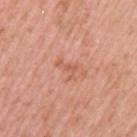Part of a total-body skin-imaging series; this lesion was reviewed on a skin check and was not flagged for biopsy. A close-up tile cropped from a whole-body skin photograph, about 15 mm across. A female patient aged 38–42. This is a white-light tile. The lesion is located on the arm. An algorithmic analysis of the crop reported a lesion area of about 2.5 mm² and two-axis asymmetry of about 0.7. The software also gave a lesion color around L≈59 a*≈27 b*≈33 in CIELAB. The software also gave a border-irregularity index near 7.5/10, internal color variation of about 0 on a 0–10 scale, and peripheral color asymmetry of about 0. It also reported a classifier nevus-likeness of about 0/100 and a detector confidence of about 100 out of 100 that the crop contains a lesion.